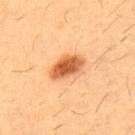Background: The lesion is located on the chest. A region of skin cropped from a whole-body photographic capture, roughly 15 mm wide. A male patient, aged 53–57.